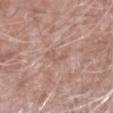Image and clinical context:
The total-body-photography lesion software estimated a color-variation rating of about 0/10 and peripheral color asymmetry of about 0. The software also gave lesion-presence confidence of about 70/100. A male patient, in their mid- to late 70s. This is a white-light tile. On the right forearm. A 15 mm close-up extracted from a 3D total-body photography capture. Longest diameter approximately 3.5 mm.